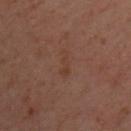Recorded during total-body skin imaging; not selected for excision or biopsy. The lesion's longest dimension is about 3 mm. On the chest. A male patient about 40 years old. This image is a 15 mm lesion crop taken from a total-body photograph. The tile uses cross-polarized illumination.